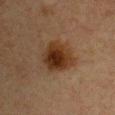On the chest. A lesion tile, about 15 mm wide, cut from a 3D total-body photograph. The patient is a female aged around 40. Captured under cross-polarized illumination. About 4.5 mm across.